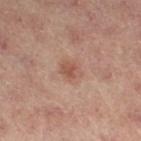| key | value |
|---|---|
| biopsy status | total-body-photography surveillance lesion; no biopsy |
| location | the left lower leg |
| patient | female, aged around 55 |
| image source | 15 mm crop, total-body photography |
| automated lesion analysis | a footprint of about 4.5 mm², an eccentricity of roughly 0.6, and a symmetry-axis asymmetry near 0.15 |
| lesion size | about 2.5 mm |